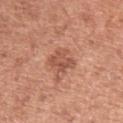Q: Is there a histopathology result?
A: no biopsy performed (imaged during a skin exam)
Q: What are the patient's age and sex?
A: female, aged approximately 50
Q: Automated lesion metrics?
A: a footprint of about 6.5 mm² and an eccentricity of roughly 0.45; an average lesion color of about L≈53 a*≈26 b*≈31 (CIELAB) and a normalized lesion–skin contrast near 6.5; border irregularity of about 3.5 on a 0–10 scale, a within-lesion color-variation index near 3/10, and peripheral color asymmetry of about 1; a classifier nevus-likeness of about 10/100 and lesion-presence confidence of about 100/100
Q: What kind of image is this?
A: ~15 mm tile from a whole-body skin photo
Q: What is the anatomic site?
A: the left upper arm
Q: What lighting was used for the tile?
A: white-light
Q: What is the lesion's diameter?
A: about 3 mm